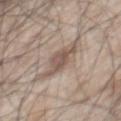Assessment: Imaged during a routine full-body skin examination; the lesion was not biopsied and no histopathology is available. Background: Longest diameter approximately 6 mm. The tile uses white-light illumination. A 15 mm close-up tile from a total-body photography series done for melanoma screening. A male patient aged 63 to 67. The lesion is on the chest.